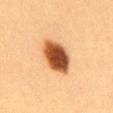Assessment: Captured during whole-body skin photography for melanoma surveillance; the lesion was not biopsied. Background: Automated tile analysis of the lesion measured a lesion area of about 13 mm² and a shape eccentricity near 0.8. The analysis additionally found a mean CIELAB color near L≈52 a*≈27 b*≈41 and a lesion-to-skin contrast of about 14 (normalized; higher = more distinct). It also reported a classifier nevus-likeness of about 100/100. Approximately 5.5 mm at its widest. A roughly 15 mm field-of-view crop from a total-body skin photograph. A female subject, aged approximately 25. The tile uses cross-polarized illumination. The lesion is located on the mid back.